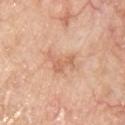<record>
<biopsy_status>not biopsied; imaged during a skin examination</biopsy_status>
<patient>
  <sex>male</sex>
  <age_approx>65</age_approx>
</patient>
<site>chest</site>
<image>
  <source>total-body photography crop</source>
  <field_of_view_mm>15</field_of_view_mm>
</image>
</record>